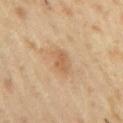Case summary:
* workup · no biopsy performed (imaged during a skin exam)
* body site · the right upper arm
* size · about 3 mm
* subject · male, aged 53 to 57
* image · ~15 mm crop, total-body skin-cancer survey
* tile lighting · cross-polarized
* automated lesion analysis · an average lesion color of about L≈57 a*≈19 b*≈35 (CIELAB), roughly 8 lightness units darker than nearby skin, and a lesion-to-skin contrast of about 6 (normalized; higher = more distinct); a border-irregularity index near 3/10, a color-variation rating of about 1/10, and peripheral color asymmetry of about 0; an automated nevus-likeness rating near 5 out of 100 and lesion-presence confidence of about 100/100